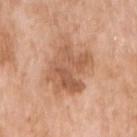size: ≈6 mm; anatomic site: the right upper arm; image: 15 mm crop, total-body photography; patient: female, aged 73–77; illumination: white-light.